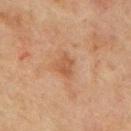No biopsy was performed on this lesion — it was imaged during a full skin examination and was not determined to be concerning. The lesion-visualizer software estimated an automated nevus-likeness rating near 0 out of 100 and a detector confidence of about 100 out of 100 that the crop contains a lesion. A male subject, aged 68–72. Measured at roughly 2.5 mm in maximum diameter. Cropped from a whole-body photographic skin survey; the tile spans about 15 mm. From the mid back. Imaged with cross-polarized lighting.